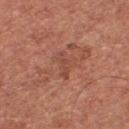image source — 15 mm crop, total-body photography | diameter — about 3.5 mm | lighting — white-light illumination | automated lesion analysis — an eccentricity of roughly 0.9 and a symmetry-axis asymmetry near 0.45; a mean CIELAB color near L≈47 a*≈25 b*≈30 and a lesion-to-skin contrast of about 4.5 (normalized; higher = more distinct) | body site — the chest | subject — male, approximately 65 years of age.